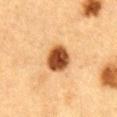biopsy status: imaged on a skin check; not biopsied | body site: the abdomen | subject: male, roughly 85 years of age or older | image source: 15 mm crop, total-body photography | lesion size: ≈3.5 mm.